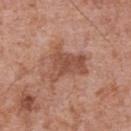Captured during whole-body skin photography for melanoma surveillance; the lesion was not biopsied. Captured under white-light illumination. The lesion-visualizer software estimated an eccentricity of roughly 0.7 and two-axis asymmetry of about 0.5. The analysis additionally found a lesion color around L≈51 a*≈23 b*≈29 in CIELAB, roughly 10 lightness units darker than nearby skin, and a lesion-to-skin contrast of about 7 (normalized; higher = more distinct). The subject is a male aged approximately 70. Cropped from a whole-body photographic skin survey; the tile spans about 15 mm. On the front of the torso.